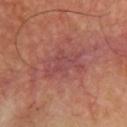Clinical impression:
Captured during whole-body skin photography for melanoma surveillance; the lesion was not biopsied.
Clinical summary:
Longest diameter approximately 5 mm. Imaged with cross-polarized lighting. On the chest. A male subject, in their mid-60s. A lesion tile, about 15 mm wide, cut from a 3D total-body photograph.